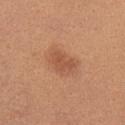Notes:
* follow-up · catalogued during a skin exam; not biopsied
* size · ≈3 mm
* tile lighting · white-light
* patient · female, roughly 25 years of age
* body site · the right upper arm
* acquisition · 15 mm crop, total-body photography